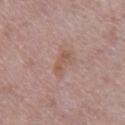• diameter · ≈3 mm
• anatomic site · the chest
• image source · ~15 mm crop, total-body skin-cancer survey
• subject · male, aged around 65
• automated lesion analysis · a normalized border contrast of about 6
• lighting · white-light